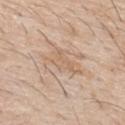Findings:
– workup · imaged on a skin check; not biopsied
– lesion diameter · ~5 mm (longest diameter)
– tile lighting · white-light
– patient · male, in their mid-50s
– image source · ~15 mm tile from a whole-body skin photo
– location · the upper back